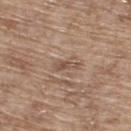Impression: No biopsy was performed on this lesion — it was imaged during a full skin examination and was not determined to be concerning. Acquisition and patient details: The lesion-visualizer software estimated border irregularity of about 4 on a 0–10 scale and a color-variation rating of about 0/10. It also reported an automated nevus-likeness rating near 0 out of 100 and a lesion-detection confidence of about 80/100. A roughly 15 mm field-of-view crop from a total-body skin photograph. The patient is a male roughly 65 years of age. From the upper back. About 3 mm across.